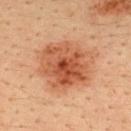Q: Is there a histopathology result?
A: catalogued during a skin exam; not biopsied
Q: What is the lesion's diameter?
A: ≈7 mm
Q: What is the anatomic site?
A: the upper back
Q: What are the patient's age and sex?
A: male, aged 33 to 37
Q: What kind of image is this?
A: total-body-photography crop, ~15 mm field of view